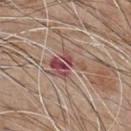Impression: Imaged during a routine full-body skin examination; the lesion was not biopsied and no histopathology is available. Acquisition and patient details: Measured at roughly 4.5 mm in maximum diameter. On the chest. The patient is a male aged approximately 65. Automated image analysis of the tile measured a footprint of about 9 mm² and an eccentricity of roughly 0.7. The analysis additionally found a lesion color around L≈49 a*≈23 b*≈22 in CIELAB and a normalized border contrast of about 8.5. A 15 mm close-up tile from a total-body photography series done for melanoma screening.